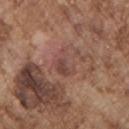Imaged during a routine full-body skin examination; the lesion was not biopsied and no histopathology is available. A region of skin cropped from a whole-body photographic capture, roughly 15 mm wide. From the chest. A male subject, aged 73–77.